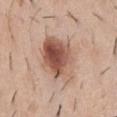No biopsy was performed on this lesion — it was imaged during a full skin examination and was not determined to be concerning. A male subject in their 40s. The lesion is located on the front of the torso. A lesion tile, about 15 mm wide, cut from a 3D total-body photograph. The tile uses white-light illumination.